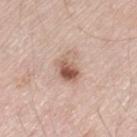biopsy status: total-body-photography surveillance lesion; no biopsy.